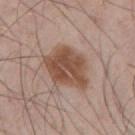biopsy status: no biopsy performed (imaged during a skin exam) | patient: male, aged 53–57 | lesion size: ~6 mm (longest diameter) | acquisition: 15 mm crop, total-body photography | illumination: white-light | anatomic site: the mid back.